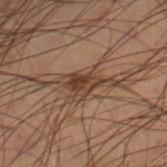Recorded during total-body skin imaging; not selected for excision or biopsy. Located on the leg. The patient is a male aged around 55. This image is a 15 mm lesion crop taken from a total-body photograph.